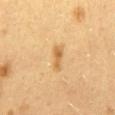{
  "biopsy_status": "not biopsied; imaged during a skin examination",
  "lesion_size": {
    "long_diameter_mm_approx": 3.0
  },
  "image": {
    "source": "total-body photography crop",
    "field_of_view_mm": 15
  },
  "site": "mid back",
  "patient": {
    "sex": "female",
    "age_approx": 40
  },
  "lighting": "cross-polarized"
}